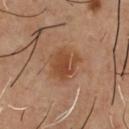This lesion was catalogued during total-body skin photography and was not selected for biopsy.
The tile uses cross-polarized illumination.
Cropped from a total-body skin-imaging series; the visible field is about 15 mm.
A male patient aged approximately 55.
The lesion is located on the chest.